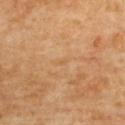Q: Is there a histopathology result?
A: total-body-photography surveillance lesion; no biopsy
Q: What is the lesion's diameter?
A: ≈1.5 mm
Q: How was the tile lit?
A: cross-polarized
Q: What are the patient's age and sex?
A: female, about 65 years old
Q: What did automated image analysis measure?
A: an average lesion color of about L≈57 a*≈19 b*≈38 (CIELAB), roughly 4 lightness units darker than nearby skin, and a normalized border contrast of about 3.5; a nevus-likeness score of about 0/100 and lesion-presence confidence of about 100/100
Q: What is the anatomic site?
A: the upper back
Q: How was this image acquired?
A: 15 mm crop, total-body photography